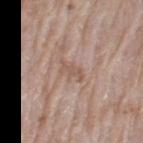Impression: Captured during whole-body skin photography for melanoma surveillance; the lesion was not biopsied. Image and clinical context: From the left thigh. A lesion tile, about 15 mm wide, cut from a 3D total-body photograph. Imaged with white-light lighting. Measured at roughly 3 mm in maximum diameter. Automated image analysis of the tile measured an automated nevus-likeness rating near 0 out of 100 and lesion-presence confidence of about 100/100. A female subject approximately 75 years of age.